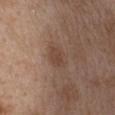Imaged during a routine full-body skin examination; the lesion was not biopsied and no histopathology is available.
A female patient, aged around 40.
On the chest.
This image is a 15 mm lesion crop taken from a total-body photograph.
The total-body-photography lesion software estimated a border-irregularity index near 3/10. And it measured an automated nevus-likeness rating near 65 out of 100.
Captured under white-light illumination.